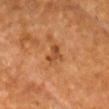Clinical impression: The lesion was tiled from a total-body skin photograph and was not biopsied. Acquisition and patient details: A male patient, about 70 years old. The lesion's longest dimension is about 2.5 mm. The total-body-photography lesion software estimated a nevus-likeness score of about 0/100 and lesion-presence confidence of about 100/100. This image is a 15 mm lesion crop taken from a total-body photograph. The lesion is on the chest. Captured under cross-polarized illumination.